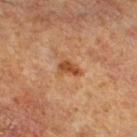| field | value |
|---|---|
| biopsy status | total-body-photography surveillance lesion; no biopsy |
| diameter | about 2.5 mm |
| subject | male, aged 63–67 |
| image | ~15 mm tile from a whole-body skin photo |
| automated metrics | a border-irregularity rating of about 3/10, a within-lesion color-variation index near 1.5/10, and a peripheral color-asymmetry measure near 0.5; an automated nevus-likeness rating near 55 out of 100 and a detector confidence of about 100 out of 100 that the crop contains a lesion |
| tile lighting | cross-polarized illumination |
| site | the right thigh |